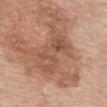Clinical impression:
Part of a total-body skin-imaging series; this lesion was reviewed on a skin check and was not flagged for biopsy.
Background:
Imaged with white-light lighting. A female subject aged around 75. From the mid back. An algorithmic analysis of the crop reported an average lesion color of about L≈52 a*≈21 b*≈30 (CIELAB), about 9 CIELAB-L* units darker than the surrounding skin, and a normalized lesion–skin contrast near 6. The software also gave a border-irregularity rating of about 8.5/10, a within-lesion color-variation index near 5/10, and radial color variation of about 1.5. A roughly 15 mm field-of-view crop from a total-body skin photograph.